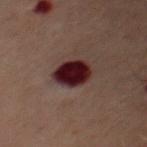Case summary:
- workup — catalogued during a skin exam; not biopsied
- imaging modality — 15 mm crop, total-body photography
- illumination — cross-polarized illumination
- location — the chest
- lesion diameter — about 4.5 mm
- patient — male, about 60 years old